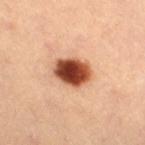The lesion was photographed on a routine skin check and not biopsied; there is no pathology result. A 15 mm crop from a total-body photograph taken for skin-cancer surveillance. The lesion is on the right thigh. Imaged with cross-polarized lighting. Measured at roughly 4 mm in maximum diameter. The patient is a female aged 43 to 47.